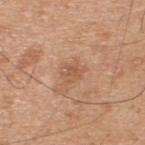Impression:
Imaged during a routine full-body skin examination; the lesion was not biopsied and no histopathology is available.
Image and clinical context:
Cropped from a total-body skin-imaging series; the visible field is about 15 mm. The subject is a male aged 43–47. The lesion is on the upper back. Longest diameter approximately 2.5 mm.